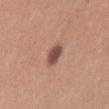<case>
  <biopsy_status>not biopsied; imaged during a skin examination</biopsy_status>
  <patient>
    <sex>male</sex>
    <age_approx>30</age_approx>
  </patient>
  <lesion_size>
    <long_diameter_mm_approx>3.0</long_diameter_mm_approx>
  </lesion_size>
  <image>
    <source>total-body photography crop</source>
    <field_of_view_mm>15</field_of_view_mm>
  </image>
  <site>back</site>
  <lighting>white-light</lighting>
</case>